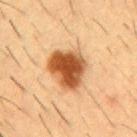biopsy status: imaged on a skin check; not biopsied
patient: male, aged around 55
imaging modality: ~15 mm tile from a whole-body skin photo
body site: the front of the torso
lesion diameter: ≈5.5 mm
illumination: cross-polarized illumination